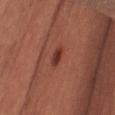No biopsy was performed on this lesion — it was imaged during a full skin examination and was not determined to be concerning.
A female subject in their 60s.
Longest diameter approximately 2.5 mm.
Captured under cross-polarized illumination.
From the right thigh.
A 15 mm close-up extracted from a 3D total-body photography capture.